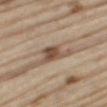Captured during whole-body skin photography for melanoma surveillance; the lesion was not biopsied.
From the right thigh.
A region of skin cropped from a whole-body photographic capture, roughly 15 mm wide.
A male subject aged 68–72.
The tile uses white-light illumination.
The recorded lesion diameter is about 4.5 mm.